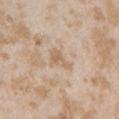Findings:
* notes · catalogued during a skin exam; not biopsied
* lesion diameter · about 3 mm
* image source · 15 mm crop, total-body photography
* location · the left upper arm
* subject · female, in their mid-20s